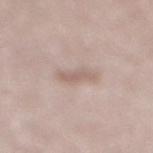Assessment: The lesion was tiled from a total-body skin photograph and was not biopsied. Image and clinical context: Located on the right lower leg. A lesion tile, about 15 mm wide, cut from a 3D total-body photograph. A female patient approximately 65 years of age.